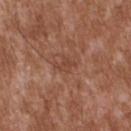Assessment: Captured during whole-body skin photography for melanoma surveillance; the lesion was not biopsied. Image and clinical context: On the upper back. A male subject, roughly 45 years of age. A region of skin cropped from a whole-body photographic capture, roughly 15 mm wide.